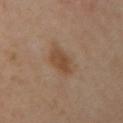Clinical impression:
The lesion was tiled from a total-body skin photograph and was not biopsied.
Clinical summary:
A female subject aged 38–42. An algorithmic analysis of the crop reported a mean CIELAB color near L≈47 a*≈18 b*≈31, a lesion–skin lightness drop of about 8, and a normalized border contrast of about 7.5. And it measured a border-irregularity rating of about 2/10, a color-variation rating of about 2.5/10, and a peripheral color-asymmetry measure near 1. And it measured a nevus-likeness score of about 70/100 and a detector confidence of about 100 out of 100 that the crop contains a lesion. On the left arm. Cropped from a total-body skin-imaging series; the visible field is about 15 mm. This is a cross-polarized tile. Approximately 3.5 mm at its widest.